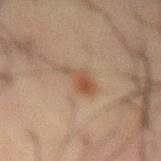notes: catalogued during a skin exam; not biopsied.